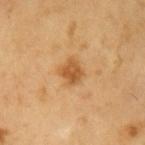notes: imaged on a skin check; not biopsied
acquisition: 15 mm crop, total-body photography
lesion diameter: ≈2.5 mm
subject: male, about 60 years old
body site: the left upper arm
tile lighting: cross-polarized
TBP lesion metrics: a border-irregularity index near 1.5/10 and a peripheral color-asymmetry measure near 1; lesion-presence confidence of about 100/100